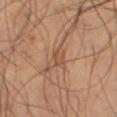biopsy_status: not biopsied; imaged during a skin examination
image:
  source: total-body photography crop
  field_of_view_mm: 15
automated_metrics:
  area_mm2_approx: 3.0
  cielab_L: 51
  cielab_a: 20
  cielab_b: 31
  vs_skin_darker_L: 8.0
  vs_skin_contrast_norm: 6.0
  border_irregularity_0_10: 3.0
  peripheral_color_asymmetry: 1.0
  nevus_likeness_0_100: 0
patient:
  sex: male
  age_approx: 65
lesion_size:
  long_diameter_mm_approx: 2.5
lighting: cross-polarized
site: left thigh